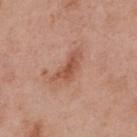Part of a total-body skin-imaging series; this lesion was reviewed on a skin check and was not flagged for biopsy. A 15 mm close-up extracted from a 3D total-body photography capture. About 4.5 mm across. The lesion is on the upper back. A male patient, approximately 55 years of age.